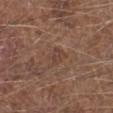The lesion is located on the left lower leg. A close-up tile cropped from a whole-body skin photograph, about 15 mm across. An algorithmic analysis of the crop reported a footprint of about 4 mm². The software also gave a mean CIELAB color near L≈42 a*≈18 b*≈26, about 6 CIELAB-L* units darker than the surrounding skin, and a lesion-to-skin contrast of about 4.5 (normalized; higher = more distinct). The analysis additionally found a border-irregularity rating of about 2.5/10 and peripheral color asymmetry of about 1. It also reported a classifier nevus-likeness of about 0/100 and a detector confidence of about 100 out of 100 that the crop contains a lesion. The tile uses white-light illumination. A male patient, about 75 years old.